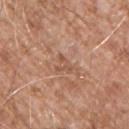<record>
<biopsy_status>not biopsied; imaged during a skin examination</biopsy_status>
<lighting>white-light</lighting>
<image>
  <source>total-body photography crop</source>
  <field_of_view_mm>15</field_of_view_mm>
</image>
<patient>
  <sex>male</sex>
  <age_approx>55</age_approx>
</patient>
<automated_metrics>
  <cielab_L>54</cielab_L>
  <cielab_a>21</cielab_a>
  <cielab_b>31</cielab_b>
  <vs_skin_darker_L>7.0</vs_skin_darker_L>
  <vs_skin_contrast_norm>5.0</vs_skin_contrast_norm>
  <border_irregularity_0_10>6.5</border_irregularity_0_10>
  <color_variation_0_10>0.0</color_variation_0_10>
  <peripheral_color_asymmetry>0.0</peripheral_color_asymmetry>
</automated_metrics>
<lesion_size>
  <long_diameter_mm_approx>2.5</long_diameter_mm_approx>
</lesion_size>
<site>left upper arm</site>
</record>